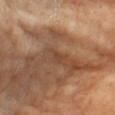Assessment:
The lesion was photographed on a routine skin check and not biopsied; there is no pathology result.
Context:
A lesion tile, about 15 mm wide, cut from a 3D total-body photograph. The tile uses cross-polarized illumination. A female patient in their mid- to late 60s. Approximately 3 mm at its widest. From the chest.